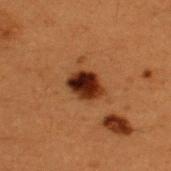Q: What lighting was used for the tile?
A: cross-polarized
Q: Who is the patient?
A: male, approximately 50 years of age
Q: Lesion size?
A: ≈3.5 mm
Q: What is the imaging modality?
A: 15 mm crop, total-body photography
Q: Lesion location?
A: the upper back